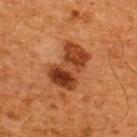Imaged during a routine full-body skin examination; the lesion was not biopsied and no histopathology is available.
A 15 mm close-up extracted from a 3D total-body photography capture.
From the upper back.
A male patient about 65 years old.